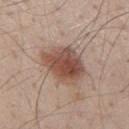Case summary:
- follow-up · imaged on a skin check; not biopsied
- subject · male, aged around 40
- anatomic site · the mid back
- image source · ~15 mm tile from a whole-body skin photo